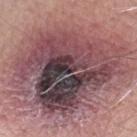Assessment:
Part of a total-body skin-imaging series; this lesion was reviewed on a skin check and was not flagged for biopsy.
Context:
A male subject aged 63–67. The lesion is located on the left forearm. A close-up tile cropped from a whole-body skin photograph, about 15 mm across.